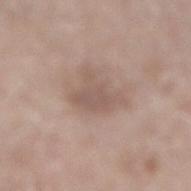Findings:
- follow-up: no biopsy performed (imaged during a skin exam)
- location: the right lower leg
- acquisition: total-body-photography crop, ~15 mm field of view
- subject: male, aged around 65
- tile lighting: white-light illumination
- size: ≈4.5 mm